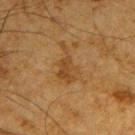{"biopsy_status": "not biopsied; imaged during a skin examination", "automated_metrics": {"eccentricity": 0.9, "vs_skin_darker_L": 6.0, "vs_skin_contrast_norm": 6.0, "nevus_likeness_0_100": 0, "lesion_detection_confidence_0_100": 100}, "site": "right upper arm", "lesion_size": {"long_diameter_mm_approx": 5.0}, "patient": {"sex": "male", "age_approx": 65}, "image": {"source": "total-body photography crop", "field_of_view_mm": 15}}